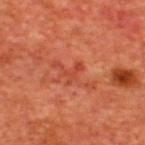biopsy status: imaged on a skin check; not biopsied | acquisition: total-body-photography crop, ~15 mm field of view | anatomic site: the upper back | image-analysis metrics: a lesion area of about 2.5 mm², an outline eccentricity of about 0.8 (0 = round, 1 = elongated), and a symmetry-axis asymmetry near 0.6; border irregularity of about 7 on a 0–10 scale, a within-lesion color-variation index near 0/10, and a peripheral color-asymmetry measure near 0; a classifier nevus-likeness of about 0/100 and a lesion-detection confidence of about 100/100 | illumination: cross-polarized illumination | subject: male, roughly 65 years of age | diameter: about 2.5 mm.